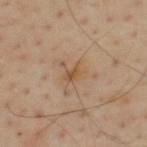A male subject in their mid-50s. Approximately 2.5 mm at its widest. The lesion is on the upper back. This is a cross-polarized tile. A 15 mm crop from a total-body photograph taken for skin-cancer surveillance.